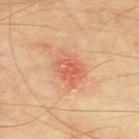Clinical impression:
Part of a total-body skin-imaging series; this lesion was reviewed on a skin check and was not flagged for biopsy.
Image and clinical context:
The lesion is on the upper back. A male patient, approximately 75 years of age. Approximately 4.5 mm at its widest. This is a cross-polarized tile. A roughly 15 mm field-of-view crop from a total-body skin photograph.